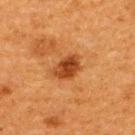The patient is a female roughly 40 years of age. On the upper back. This is a cross-polarized tile. A 15 mm close-up extracted from a 3D total-body photography capture.